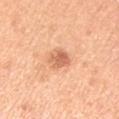Impression: The lesion was tiled from a total-body skin photograph and was not biopsied. Image and clinical context: A close-up tile cropped from a whole-body skin photograph, about 15 mm across. A male subject approximately 50 years of age. Longest diameter approximately 2.5 mm. Located on the left upper arm.